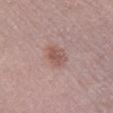{
  "lesion_size": {
    "long_diameter_mm_approx": 3.0
  },
  "image": {
    "source": "total-body photography crop",
    "field_of_view_mm": 15
  },
  "site": "leg",
  "patient": {
    "sex": "female",
    "age_approx": 40
  },
  "automated_metrics": {
    "nevus_likeness_0_100": 30,
    "lesion_detection_confidence_0_100": 100
  }
}